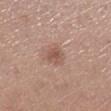Captured during whole-body skin photography for melanoma surveillance; the lesion was not biopsied. The patient is a female aged 38–42. Located on the leg. A region of skin cropped from a whole-body photographic capture, roughly 15 mm wide.